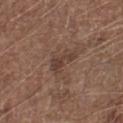Q: Was a biopsy performed?
A: no biopsy performed (imaged during a skin exam)
Q: What is the imaging modality?
A: ~15 mm crop, total-body skin-cancer survey
Q: Automated lesion metrics?
A: a lesion area of about 4 mm², an outline eccentricity of about 0.9 (0 = round, 1 = elongated), and a shape-asymmetry score of about 0.55 (0 = symmetric); a nevus-likeness score of about 0/100 and lesion-presence confidence of about 100/100
Q: What are the patient's age and sex?
A: male, aged around 75
Q: Illumination type?
A: white-light
Q: Lesion location?
A: the left lower leg